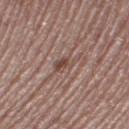Assessment:
Recorded during total-body skin imaging; not selected for excision or biopsy.
Context:
From the right thigh. A roughly 15 mm field-of-view crop from a total-body skin photograph. The patient is a female about 50 years old.